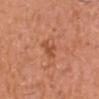Q: Was this lesion biopsied?
A: total-body-photography surveillance lesion; no biopsy
Q: What are the patient's age and sex?
A: male, aged 38–42
Q: What is the imaging modality?
A: 15 mm crop, total-body photography
Q: What is the lesion's diameter?
A: about 3 mm
Q: Lesion location?
A: the head or neck
Q: Illumination type?
A: white-light
Q: Automated lesion metrics?
A: a lesion area of about 3.5 mm² and an outline eccentricity of about 0.9 (0 = round, 1 = elongated); a lesion color around L≈51 a*≈28 b*≈36 in CIELAB and a lesion-to-skin contrast of about 6.5 (normalized; higher = more distinct); a classifier nevus-likeness of about 0/100 and a lesion-detection confidence of about 100/100